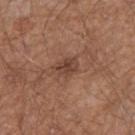Part of a total-body skin-imaging series; this lesion was reviewed on a skin check and was not flagged for biopsy.
A male patient approximately 55 years of age.
A 15 mm close-up extracted from a 3D total-body photography capture.
Located on the arm.